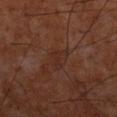<tbp_lesion>
  <biopsy_status>not biopsied; imaged during a skin examination</biopsy_status>
  <lesion_size>
    <long_diameter_mm_approx>3.0</long_diameter_mm_approx>
  </lesion_size>
  <lighting>cross-polarized</lighting>
  <patient>
    <sex>male</sex>
    <age_approx>60</age_approx>
  </patient>
  <image>
    <source>total-body photography crop</source>
    <field_of_view_mm>15</field_of_view_mm>
  </image>
  <site>upper back</site>
</tbp_lesion>